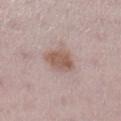<record>
<biopsy_status>not biopsied; imaged during a skin examination</biopsy_status>
<lesion_size>
  <long_diameter_mm_approx>3.5</long_diameter_mm_approx>
</lesion_size>
<lighting>white-light</lighting>
<patient>
  <sex>female</sex>
  <age_approx>30</age_approx>
</patient>
<site>leg</site>
<automated_metrics>
  <area_mm2_approx>8.0</area_mm2_approx>
  <shape_asymmetry>0.15</shape_asymmetry>
  <cielab_L>56</cielab_L>
  <cielab_a>18</cielab_a>
  <cielab_b>24</cielab_b>
  <vs_skin_darker_L>10.0</vs_skin_darker_L>
  <vs_skin_contrast_norm>8.0</vs_skin_contrast_norm>
  <nevus_likeness_0_100>65</nevus_likeness_0_100>
  <lesion_detection_confidence_0_100>100</lesion_detection_confidence_0_100>
</automated_metrics>
<image>
  <source>total-body photography crop</source>
  <field_of_view_mm>15</field_of_view_mm>
</image>
</record>